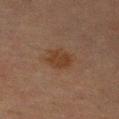| field | value |
|---|---|
| follow-up | total-body-photography surveillance lesion; no biopsy |
| body site | the left upper arm |
| imaging modality | total-body-photography crop, ~15 mm field of view |
| patient | male, in their mid- to late 60s |